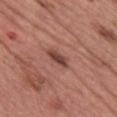The lesion was photographed on a routine skin check and not biopsied; there is no pathology result. The patient is a female aged 58–62. A roughly 15 mm field-of-view crop from a total-body skin photograph. Captured under white-light illumination. On the chest.